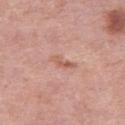The lesion was photographed on a routine skin check and not biopsied; there is no pathology result.
A female patient, aged around 40.
A 15 mm close-up extracted from a 3D total-body photography capture.
The lesion is located on the left thigh.
Longest diameter approximately 3.5 mm.